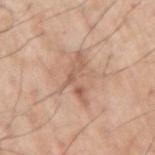Imaged during a routine full-body skin examination; the lesion was not biopsied and no histopathology is available. A male subject, aged 68–72. Captured under white-light illumination. An algorithmic analysis of the crop reported a nevus-likeness score of about 0/100 and a lesion-detection confidence of about 95/100. Located on the right upper arm. A roughly 15 mm field-of-view crop from a total-body skin photograph. The recorded lesion diameter is about 5.5 mm.